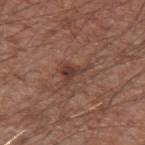{"biopsy_status": "not biopsied; imaged during a skin examination", "automated_metrics": {"cielab_L": 39, "cielab_a": 20, "cielab_b": 24, "vs_skin_darker_L": 8.0, "vs_skin_contrast_norm": 7.5, "border_irregularity_0_10": 7.0, "peripheral_color_asymmetry": 0.5}, "site": "left upper arm", "lighting": "white-light", "patient": {"sex": "male", "age_approx": 75}, "image": {"source": "total-body photography crop", "field_of_view_mm": 15}}